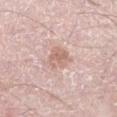• notes — total-body-photography surveillance lesion; no biopsy
• anatomic site — the left lower leg
• subject — male, roughly 50 years of age
• diameter — ~4 mm (longest diameter)
• image — 15 mm crop, total-body photography